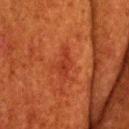The lesion was photographed on a routine skin check and not biopsied; there is no pathology result. Located on the head or neck. Measured at roughly 3 mm in maximum diameter. Cropped from a whole-body photographic skin survey; the tile spans about 15 mm. The total-body-photography lesion software estimated a lesion–skin lightness drop of about 6 and a normalized lesion–skin contrast near 6. The analysis additionally found border irregularity of about 5 on a 0–10 scale and peripheral color asymmetry of about 0.5. The patient is a male approximately 60 years of age. Captured under cross-polarized illumination.